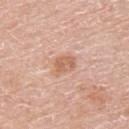Captured during whole-body skin photography for melanoma surveillance; the lesion was not biopsied. Automated tile analysis of the lesion measured a footprint of about 5 mm², an outline eccentricity of about 0.65 (0 = round, 1 = elongated), and a shape-asymmetry score of about 0.25 (0 = symmetric). It also reported border irregularity of about 2 on a 0–10 scale and a peripheral color-asymmetry measure near 1. A 15 mm close-up extracted from a 3D total-body photography capture. This is a white-light tile. About 3 mm across. From the upper back. A male subject, about 60 years old.